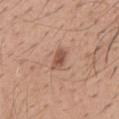No biopsy was performed on this lesion — it was imaged during a full skin examination and was not determined to be concerning.
A 15 mm close-up extracted from a 3D total-body photography capture.
The lesion is located on the mid back.
Automated image analysis of the tile measured an average lesion color of about L≈53 a*≈22 b*≈29 (CIELAB) and a normalized lesion–skin contrast near 8. The software also gave a border-irregularity index near 2/10, internal color variation of about 3 on a 0–10 scale, and radial color variation of about 1. And it measured an automated nevus-likeness rating near 85 out of 100 and a lesion-detection confidence of about 100/100.
A male patient about 40 years old.
The recorded lesion diameter is about 3 mm.
This is a white-light tile.